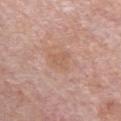  biopsy_status: not biopsied; imaged during a skin examination
  patient:
    sex: male
    age_approx: 55
  image:
    source: total-body photography crop
    field_of_view_mm: 15
  site: chest
  lesion_size:
    long_diameter_mm_approx: 3.0